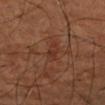Assessment: The lesion was tiled from a total-body skin photograph and was not biopsied. Image and clinical context: Automated tile analysis of the lesion measured a lesion color around L≈33 a*≈22 b*≈28 in CIELAB and a lesion–skin lightness drop of about 5. Imaged with cross-polarized lighting. A 15 mm close-up extracted from a 3D total-body photography capture. A subject in their mid-60s. The recorded lesion diameter is about 3 mm. The lesion is on the left forearm.